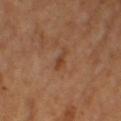Q: Was this lesion biopsied?
A: no biopsy performed (imaged during a skin exam)
Q: What is the imaging modality?
A: 15 mm crop, total-body photography
Q: What are the patient's age and sex?
A: female, in their mid- to late 50s
Q: Automated lesion metrics?
A: a border-irregularity index near 3/10, a color-variation rating of about 0/10, and radial color variation of about 0; a classifier nevus-likeness of about 0/100
Q: Illumination type?
A: cross-polarized illumination
Q: How large is the lesion?
A: ~2.5 mm (longest diameter)
Q: Where on the body is the lesion?
A: the right thigh